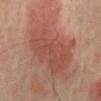Captured during whole-body skin photography for melanoma surveillance; the lesion was not biopsied. The tile uses cross-polarized illumination. The recorded lesion diameter is about 12 mm. The lesion is located on the front of the torso. A male subject, aged 63–67. Cropped from a whole-body photographic skin survey; the tile spans about 15 mm.